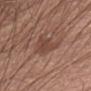Notes:
• follow-up — catalogued during a skin exam; not biopsied
• size — ~4 mm (longest diameter)
• imaging modality — 15 mm crop, total-body photography
• tile lighting — white-light
• patient — male, aged 63 to 67
• image-analysis metrics — a border-irregularity index near 4/10, a within-lesion color-variation index near 2.5/10, and peripheral color asymmetry of about 1; an automated nevus-likeness rating near 10 out of 100 and a lesion-detection confidence of about 100/100
• body site — the chest